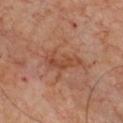Part of a total-body skin-imaging series; this lesion was reviewed on a skin check and was not flagged for biopsy.
The lesion is on the chest.
A male patient, aged approximately 70.
Captured under cross-polarized illumination.
A roughly 15 mm field-of-view crop from a total-body skin photograph.
Automated tile analysis of the lesion measured a within-lesion color-variation index near 3.5/10.